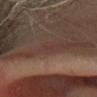workup = catalogued during a skin exam; not biopsied | subject = female, aged 58–62 | body site = the head or neck | acquisition = ~15 mm crop, total-body skin-cancer survey | size = about 1.5 mm | tile lighting = cross-polarized illumination.